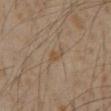Findings:
- follow-up · catalogued during a skin exam; not biopsied
- acquisition · total-body-photography crop, ~15 mm field of view
- automated metrics · roughly 6 lightness units darker than nearby skin and a normalized border contrast of about 5.5
- patient · male, in their 60s
- anatomic site · the front of the torso
- size · about 3 mm